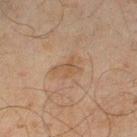| feature | finding |
|---|---|
| workup | total-body-photography surveillance lesion; no biopsy |
| acquisition | 15 mm crop, total-body photography |
| illumination | cross-polarized |
| automated lesion analysis | an area of roughly 5 mm², an eccentricity of roughly 0.8, and two-axis asymmetry of about 0.4; a classifier nevus-likeness of about 0/100 and a detector confidence of about 100 out of 100 that the crop contains a lesion |
| site | the leg |
| patient | male, in their mid-40s |
| lesion size | ≈3.5 mm |